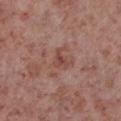<record>
  <biopsy_status>not biopsied; imaged during a skin examination</biopsy_status>
  <lighting>white-light</lighting>
  <automated_metrics>
    <area_mm2_approx>3.5</area_mm2_approx>
    <eccentricity>0.6</eccentricity>
    <shape_asymmetry>0.5</shape_asymmetry>
    <border_irregularity_0_10>5.0</border_irregularity_0_10>
    <peripheral_color_asymmetry>1.0</peripheral_color_asymmetry>
    <nevus_likeness_0_100>0</nevus_likeness_0_100>
    <lesion_detection_confidence_0_100>100</lesion_detection_confidence_0_100>
  </automated_metrics>
  <site>leg</site>
  <patient>
    <sex>male</sex>
    <age_approx>55</age_approx>
  </patient>
  <image>
    <source>total-body photography crop</source>
    <field_of_view_mm>15</field_of_view_mm>
  </image>
</record>